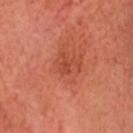Captured during whole-body skin photography for melanoma surveillance; the lesion was not biopsied. On the head or neck. This is a cross-polarized tile. A 15 mm close-up tile from a total-body photography series done for melanoma screening. A male subject roughly 65 years of age.